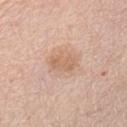Q: Patient demographics?
A: female, aged around 75
Q: How large is the lesion?
A: about 3.5 mm
Q: What kind of image is this?
A: 15 mm crop, total-body photography
Q: Where on the body is the lesion?
A: the abdomen
Q: What lighting was used for the tile?
A: white-light illumination